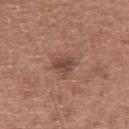biopsy status: total-body-photography surveillance lesion; no biopsy
subject: male, aged 58 to 62
image: total-body-photography crop, ~15 mm field of view
body site: the upper back
lighting: white-light